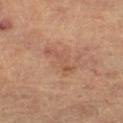Recorded during total-body skin imaging; not selected for excision or biopsy.